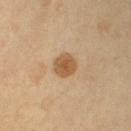Notes:
* biopsy status · no biopsy performed (imaged during a skin exam)
* body site · the left upper arm
* patient · male, aged approximately 55
* image · ~15 mm tile from a whole-body skin photo
* size · ≈3 mm
* illumination · cross-polarized illumination